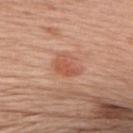biopsy status = total-body-photography surveillance lesion; no biopsy
image source = ~15 mm tile from a whole-body skin photo
lesion diameter = ≈3.5 mm
body site = the upper back
automated lesion analysis = a nevus-likeness score of about 80/100 and lesion-presence confidence of about 100/100
subject = female, approximately 60 years of age
illumination = white-light illumination